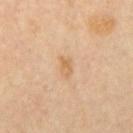Clinical impression:
Imaged during a routine full-body skin examination; the lesion was not biopsied and no histopathology is available.